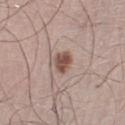Assessment: No biopsy was performed on this lesion — it was imaged during a full skin examination and was not determined to be concerning.